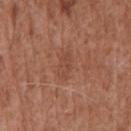<case>
<lesion_size>
  <long_diameter_mm_approx>3.0</long_diameter_mm_approx>
</lesion_size>
<patient>
  <sex>male</sex>
  <age_approx>75</age_approx>
</patient>
<site>chest</site>
<automated_metrics>
  <area_mm2_approx>3.0</area_mm2_approx>
  <eccentricity>0.9</eccentricity>
  <shape_asymmetry>0.35</shape_asymmetry>
  <cielab_L>46</cielab_L>
  <cielab_a>23</cielab_a>
  <cielab_b>30</cielab_b>
  <vs_skin_darker_L>6.0</vs_skin_darker_L>
  <vs_skin_contrast_norm>4.5</vs_skin_contrast_norm>
  <nevus_likeness_0_100>0</nevus_likeness_0_100>
  <lesion_detection_confidence_0_100>100</lesion_detection_confidence_0_100>
</automated_metrics>
<lighting>white-light</lighting>
<image>
  <source>total-body photography crop</source>
  <field_of_view_mm>15</field_of_view_mm>
</image>
</case>